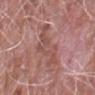Assessment: The lesion was tiled from a total-body skin photograph and was not biopsied. Image and clinical context: The lesion is on the right forearm. The patient is a male about 75 years old. A lesion tile, about 15 mm wide, cut from a 3D total-body photograph.